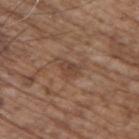Notes:
• follow-up: imaged on a skin check; not biopsied
• patient: male, aged around 70
• location: the upper back
• lighting: white-light
• image: ~15 mm tile from a whole-body skin photo